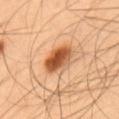No biopsy was performed on this lesion — it was imaged during a full skin examination and was not determined to be concerning. This is a cross-polarized tile. A lesion tile, about 15 mm wide, cut from a 3D total-body photograph. A male subject, aged approximately 55. Approximately 4.5 mm at its widest. The lesion is on the mid back.